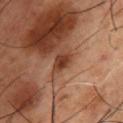Clinical impression: Recorded during total-body skin imaging; not selected for excision or biopsy. Context: A 15 mm crop from a total-body photograph taken for skin-cancer surveillance. On the front of the torso. Captured under cross-polarized illumination. A male subject, aged 53–57.